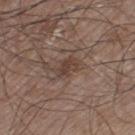Part of a total-body skin-imaging series; this lesion was reviewed on a skin check and was not flagged for biopsy. Approximately 3 mm at its widest. The patient is a male aged around 60. On the left thigh. A region of skin cropped from a whole-body photographic capture, roughly 15 mm wide.